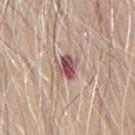biopsy status — catalogued during a skin exam; not biopsied | image source — ~15 mm tile from a whole-body skin photo | patient — male, aged approximately 65 | site — the mid back | automated lesion analysis — a mean CIELAB color near L≈54 a*≈22 b*≈21, about 14 CIELAB-L* units darker than the surrounding skin, and a lesion-to-skin contrast of about 10 (normalized; higher = more distinct); a border-irregularity index near 3.5/10, a color-variation rating of about 9/10, and radial color variation of about 3; a nevus-likeness score of about 0/100 and lesion-presence confidence of about 100/100 | lighting — white-light illumination | lesion diameter — ≈3.5 mm.